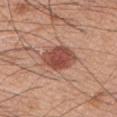Recorded during total-body skin imaging; not selected for excision or biopsy. The patient is a male approximately 60 years of age. The total-body-photography lesion software estimated an area of roughly 12 mm², a shape eccentricity near 0.7, and a shape-asymmetry score of about 0.15 (0 = symmetric). It also reported internal color variation of about 4 on a 0–10 scale and radial color variation of about 1. The software also gave a nevus-likeness score of about 95/100 and lesion-presence confidence of about 100/100. The lesion is located on the left upper arm. A 15 mm crop from a total-body photograph taken for skin-cancer surveillance. This is a white-light tile. Measured at roughly 5 mm in maximum diameter.